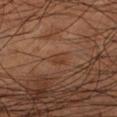{
  "biopsy_status": "not biopsied; imaged during a skin examination",
  "site": "left lower leg",
  "automated_metrics": {
    "nevus_likeness_0_100": 0,
    "lesion_detection_confidence_0_100": 100
  },
  "lighting": "cross-polarized",
  "image": {
    "source": "total-body photography crop",
    "field_of_view_mm": 15
  },
  "patient": {
    "sex": "male",
    "age_approx": 55
  }
}